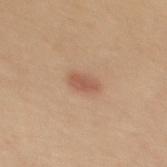follow-up: total-body-photography surveillance lesion; no biopsy | body site: the upper back | patient: female, aged around 30 | diameter: ≈3 mm | image: ~15 mm tile from a whole-body skin photo.